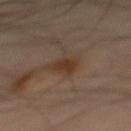follow-up = total-body-photography surveillance lesion; no biopsy | body site = the mid back | lighting = cross-polarized | size = about 3 mm | subject = male, roughly 50 years of age | image = total-body-photography crop, ~15 mm field of view.